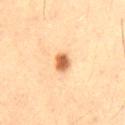<record>
  <biopsy_status>not biopsied; imaged during a skin examination</biopsy_status>
  <patient>
    <sex>male</sex>
    <age_approx>50</age_approx>
  </patient>
  <site>right thigh</site>
  <image>
    <source>total-body photography crop</source>
    <field_of_view_mm>15</field_of_view_mm>
  </image>
  <lesion_size>
    <long_diameter_mm_approx>2.5</long_diameter_mm_approx>
  </lesion_size>
  <lighting>cross-polarized</lighting>
</record>